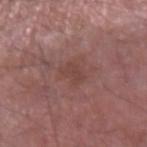Case summary:
- notes — imaged on a skin check; not biopsied
- subject — male, aged approximately 65
- lighting — white-light illumination
- body site — the right forearm
- image-analysis metrics — a lesion area of about 4 mm² and a shape eccentricity near 0.85; an average lesion color of about L≈43 a*≈21 b*≈23 (CIELAB), a lesion–skin lightness drop of about 6, and a lesion-to-skin contrast of about 5 (normalized; higher = more distinct); border irregularity of about 4 on a 0–10 scale, a within-lesion color-variation index near 1.5/10, and a peripheral color-asymmetry measure near 0.5; a classifier nevus-likeness of about 0/100
- image source — total-body-photography crop, ~15 mm field of view
- size — ≈3 mm